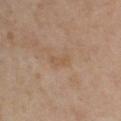follow-up=catalogued during a skin exam; not biopsied
anatomic site=the left upper arm
imaging modality=~15 mm crop, total-body skin-cancer survey
subject=female, about 50 years old
lesion size=~3 mm (longest diameter)